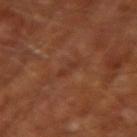workup: imaged on a skin check; not biopsied | image: total-body-photography crop, ~15 mm field of view | lesion diameter: about 2.5 mm | tile lighting: cross-polarized | TBP lesion metrics: a footprint of about 2.5 mm², an eccentricity of roughly 0.9, and a shape-asymmetry score of about 0.3 (0 = symmetric); border irregularity of about 4 on a 0–10 scale and a within-lesion color-variation index near 0/10 | subject: male, in their mid-60s | body site: the right upper arm.